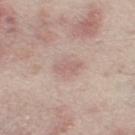Case summary:
* biopsy status · total-body-photography surveillance lesion; no biopsy
* automated lesion analysis · a mean CIELAB color near L≈62 a*≈18 b*≈22, a lesion–skin lightness drop of about 7, and a normalized border contrast of about 4.5; border irregularity of about 2.5 on a 0–10 scale and a color-variation rating of about 1.5/10
* illumination · white-light
* lesion size · ≈3 mm
* acquisition · ~15 mm tile from a whole-body skin photo
* location · the leg
* patient · male, aged 63–67